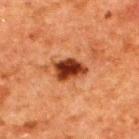This lesion was catalogued during total-body skin photography and was not selected for biopsy. On the upper back. A roughly 15 mm field-of-view crop from a total-body skin photograph. A male subject aged 58 to 62.